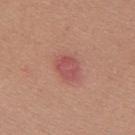The lesion was tiled from a total-body skin photograph and was not biopsied. A female patient roughly 25 years of age. From the upper back. Automated tile analysis of the lesion measured an outline eccentricity of about 0.65 (0 = round, 1 = elongated) and a shape-asymmetry score of about 0.15 (0 = symmetric). The software also gave a border-irregularity rating of about 1.5/10 and a within-lesion color-variation index near 2/10. Cropped from a whole-body photographic skin survey; the tile spans about 15 mm.